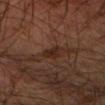Acquisition and patient details: The total-body-photography lesion software estimated an area of roughly 4 mm² and a symmetry-axis asymmetry near 0.3. And it measured a lesion color around L≈25 a*≈17 b*≈23 in CIELAB and about 7 CIELAB-L* units darker than the surrounding skin. The software also gave a border-irregularity index near 3/10, a color-variation rating of about 1/10, and a peripheral color-asymmetry measure near 0.5. A male subject, aged 63–67. A 15 mm close-up extracted from a 3D total-body photography capture. The lesion's longest dimension is about 3 mm. From the left forearm. This is a cross-polarized tile.